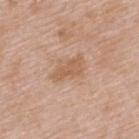follow-up=imaged on a skin check; not biopsied | subject=male, in their mid-60s | lighting=white-light | lesion diameter=~4 mm (longest diameter) | image-analysis metrics=a normalized lesion–skin contrast near 6.5; a border-irregularity rating of about 4.5/10, a within-lesion color-variation index near 1.5/10, and a peripheral color-asymmetry measure near 0.5 | body site=the upper back | imaging modality=~15 mm crop, total-body skin-cancer survey.